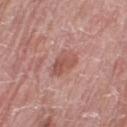| key | value |
|---|---|
| biopsy status | total-body-photography surveillance lesion; no biopsy |
| image-analysis metrics | an outline eccentricity of about 0.75 (0 = round, 1 = elongated) and a symmetry-axis asymmetry near 0.25; a lesion–skin lightness drop of about 9 and a lesion-to-skin contrast of about 6.5 (normalized; higher = more distinct); a classifier nevus-likeness of about 25/100 and a detector confidence of about 100 out of 100 that the crop contains a lesion |
| subject | female, in their 60s |
| imaging modality | ~15 mm crop, total-body skin-cancer survey |
| site | the right thigh |